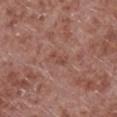Clinical summary:
The lesion's longest dimension is about 2.5 mm. The tile uses white-light illumination. Cropped from a total-body skin-imaging series; the visible field is about 15 mm. The lesion is on the left lower leg. The subject is a male aged 53 to 57.